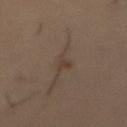Clinical impression:
Part of a total-body skin-imaging series; this lesion was reviewed on a skin check and was not flagged for biopsy.
Context:
A lesion tile, about 15 mm wide, cut from a 3D total-body photograph. On the abdomen. A male patient, aged 53–57.